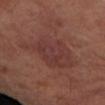Imaged during a routine full-body skin examination; the lesion was not biopsied and no histopathology is available.
A male subject, aged around 70.
This is a cross-polarized tile.
This image is a 15 mm lesion crop taken from a total-body photograph.
The lesion is on the arm.
The lesion's longest dimension is about 6.5 mm.